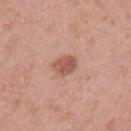This lesion was catalogued during total-body skin photography and was not selected for biopsy. Measured at roughly 2.5 mm in maximum diameter. The subject is a female aged 38 to 42. A lesion tile, about 15 mm wide, cut from a 3D total-body photograph. From the left upper arm. The lesion-visualizer software estimated a footprint of about 5 mm², an eccentricity of roughly 0.65, and a symmetry-axis asymmetry near 0.2. It also reported an average lesion color of about L≈55 a*≈24 b*≈28 (CIELAB) and a normalized lesion–skin contrast near 7.5.